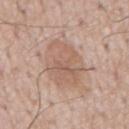<tbp_lesion>
  <biopsy_status>not biopsied; imaged during a skin examination</biopsy_status>
  <lesion_size>
    <long_diameter_mm_approx>6.5</long_diameter_mm_approx>
  </lesion_size>
  <patient>
    <sex>male</sex>
    <age_approx>60</age_approx>
  </patient>
  <image>
    <source>total-body photography crop</source>
    <field_of_view_mm>15</field_of_view_mm>
  </image>
  <site>front of the torso</site>
</tbp_lesion>